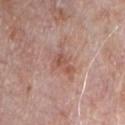No biopsy was performed on this lesion — it was imaged during a full skin examination and was not determined to be concerning. The lesion is on the chest. A male patient in their 70s. Measured at roughly 4.5 mm in maximum diameter. A roughly 15 mm field-of-view crop from a total-body skin photograph. Captured under white-light illumination.